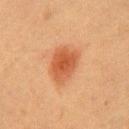{
  "biopsy_status": "not biopsied; imaged during a skin examination",
  "lighting": "cross-polarized",
  "lesion_size": {
    "long_diameter_mm_approx": 4.5
  },
  "image": {
    "source": "total-body photography crop",
    "field_of_view_mm": 15
  },
  "site": "chest",
  "automated_metrics": {
    "nevus_likeness_0_100": 100,
    "lesion_detection_confidence_0_100": 100
  },
  "patient": {
    "sex": "female",
    "age_approx": 50
  }
}